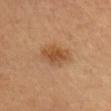{
  "biopsy_status": "not biopsied; imaged during a skin examination",
  "patient": {
    "sex": "female",
    "age_approx": 45
  },
  "lesion_size": {
    "long_diameter_mm_approx": 4.0
  },
  "lighting": "cross-polarized",
  "site": "head or neck",
  "image": {
    "source": "total-body photography crop",
    "field_of_view_mm": 15
  }
}